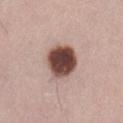Q: Was this lesion biopsied?
A: imaged on a skin check; not biopsied
Q: What is the lesion's diameter?
A: about 4.5 mm
Q: What is the anatomic site?
A: the left thigh
Q: What is the imaging modality?
A: 15 mm crop, total-body photography
Q: Illumination type?
A: white-light
Q: Automated lesion metrics?
A: a nevus-likeness score of about 70/100 and a detector confidence of about 100 out of 100 that the crop contains a lesion
Q: What are the patient's age and sex?
A: male, in their mid-40s